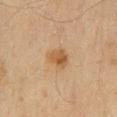notes=imaged on a skin check; not biopsied | image source=~15 mm crop, total-body skin-cancer survey | size=≈3 mm | patient=male, aged 43–47 | body site=the back.